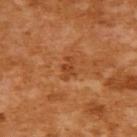Recorded during total-body skin imaging; not selected for excision or biopsy. Cropped from a whole-body photographic skin survey; the tile spans about 15 mm. Measured at roughly 2.5 mm in maximum diameter. The subject is a female aged 53 to 57. The lesion is on the back.